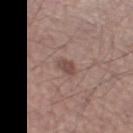The lesion was tiled from a total-body skin photograph and was not biopsied. The subject is a male approximately 55 years of age. Cropped from a total-body skin-imaging series; the visible field is about 15 mm. Imaged with white-light lighting. On the right lower leg.